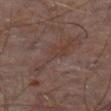| feature | finding |
|---|---|
| biopsy status | total-body-photography surveillance lesion; no biopsy |
| patient | male, aged around 60 |
| illumination | cross-polarized |
| image source | 15 mm crop, total-body photography |
| body site | the abdomen |
| lesion diameter | ≈8.5 mm |